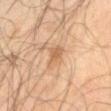Imaged during a routine full-body skin examination; the lesion was not biopsied and no histopathology is available. On the right upper arm. The patient is a male in their mid-60s. A 15 mm close-up tile from a total-body photography series done for melanoma screening.